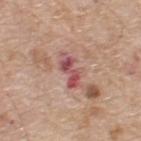- biopsy status — no biopsy performed (imaged during a skin exam)
- body site — the back
- patient — male, aged around 70
- image source — ~15 mm crop, total-body skin-cancer survey
- automated metrics — an outline eccentricity of about 0.85 (0 = round, 1 = elongated); border irregularity of about 5.5 on a 0–10 scale and a peripheral color-asymmetry measure near 1.5; an automated nevus-likeness rating near 0 out of 100 and a detector confidence of about 100 out of 100 that the crop contains a lesion
- illumination — white-light